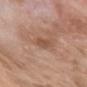Assessment: Captured during whole-body skin photography for melanoma surveillance; the lesion was not biopsied. Context: On the head or neck. This is a white-light tile. Measured at roughly 3 mm in maximum diameter. An algorithmic analysis of the crop reported an average lesion color of about L≈51 a*≈21 b*≈30 (CIELAB), roughly 8 lightness units darker than nearby skin, and a normalized border contrast of about 6. And it measured border irregularity of about 2 on a 0–10 scale, a within-lesion color-variation index near 2.5/10, and a peripheral color-asymmetry measure near 1. A roughly 15 mm field-of-view crop from a total-body skin photograph. A male patient aged 78–82.